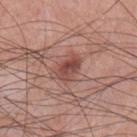Captured during whole-body skin photography for melanoma surveillance; the lesion was not biopsied.
A male subject aged around 75.
On the chest.
A 15 mm crop from a total-body photograph taken for skin-cancer surveillance.